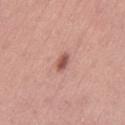Q: Is there a histopathology result?
A: total-body-photography surveillance lesion; no biopsy
Q: Lesion location?
A: the left thigh
Q: What kind of image is this?
A: 15 mm crop, total-body photography
Q: What are the patient's age and sex?
A: female, roughly 35 years of age
Q: How was the tile lit?
A: white-light